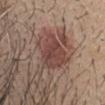Q: Was a biopsy performed?
A: catalogued during a skin exam; not biopsied
Q: What is the imaging modality?
A: ~15 mm tile from a whole-body skin photo
Q: What is the lesion's diameter?
A: about 4.5 mm
Q: Illumination type?
A: white-light
Q: What are the patient's age and sex?
A: male, approximately 30 years of age
Q: Lesion location?
A: the head or neck